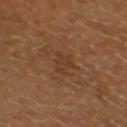The subject is a male aged approximately 60. On the head or neck. A 15 mm crop from a total-body photograph taken for skin-cancer surveillance. The tile uses cross-polarized illumination. Automated tile analysis of the lesion measured an average lesion color of about L≈34 a*≈18 b*≈29 (CIELAB), a lesion–skin lightness drop of about 5, and a normalized lesion–skin contrast near 5. And it measured a border-irregularity rating of about 4.5/10 and a within-lesion color-variation index near 2/10. The recorded lesion diameter is about 3.5 mm.